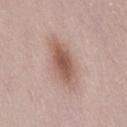This lesion was catalogued during total-body skin photography and was not selected for biopsy. A 15 mm close-up tile from a total-body photography series done for melanoma screening. An algorithmic analysis of the crop reported a lesion area of about 14 mm², an eccentricity of roughly 0.85, and a symmetry-axis asymmetry near 0.15. It also reported a mean CIELAB color near L≈57 a*≈19 b*≈26, roughly 12 lightness units darker than nearby skin, and a normalized lesion–skin contrast near 8. It also reported internal color variation of about 5 on a 0–10 scale and radial color variation of about 1.5. It also reported a nevus-likeness score of about 100/100. The lesion is on the lower back. The subject is a female aged 48 to 52.